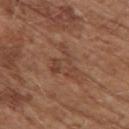notes: imaged on a skin check; not biopsied | acquisition: total-body-photography crop, ~15 mm field of view | location: the upper back | subject: male, about 75 years old.